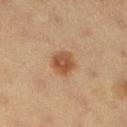The lesion was tiled from a total-body skin photograph and was not biopsied.
On the leg.
Automated image analysis of the tile measured a footprint of about 6.5 mm², a shape eccentricity near 0.55, and a shape-asymmetry score of about 0.2 (0 = symmetric). The software also gave border irregularity of about 2 on a 0–10 scale, internal color variation of about 4 on a 0–10 scale, and peripheral color asymmetry of about 1.5. It also reported a classifier nevus-likeness of about 100/100 and a lesion-detection confidence of about 100/100.
A female patient aged around 50.
A region of skin cropped from a whole-body photographic capture, roughly 15 mm wide.
The lesion's longest dimension is about 3 mm.
Imaged with cross-polarized lighting.